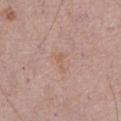Part of a total-body skin-imaging series; this lesion was reviewed on a skin check and was not flagged for biopsy. A lesion tile, about 15 mm wide, cut from a 3D total-body photograph. The lesion is on the front of the torso. The patient is a male aged 68–72.